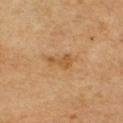This lesion was catalogued during total-body skin photography and was not selected for biopsy.
On the chest.
A region of skin cropped from a whole-body photographic capture, roughly 15 mm wide.
Captured under cross-polarized illumination.
A male subject aged 58 to 62.
About 3 mm across.
The lesion-visualizer software estimated about 8 CIELAB-L* units darker than the surrounding skin and a normalized border contrast of about 6.5.